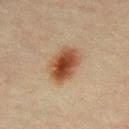Recorded during total-body skin imaging; not selected for excision or biopsy. A male subject about 50 years old. About 5 mm across. The tile uses cross-polarized illumination. A region of skin cropped from a whole-body photographic capture, roughly 15 mm wide. Located on the mid back. Automated tile analysis of the lesion measured two-axis asymmetry of about 0.15. The analysis additionally found a border-irregularity rating of about 2/10, internal color variation of about 6.5 on a 0–10 scale, and peripheral color asymmetry of about 2. The software also gave a nevus-likeness score of about 100/100 and a detector confidence of about 100 out of 100 that the crop contains a lesion.